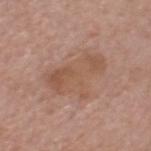{
  "biopsy_status": "not biopsied; imaged during a skin examination",
  "site": "head or neck",
  "patient": {
    "sex": "male",
    "age_approx": 65
  },
  "image": {
    "source": "total-body photography crop",
    "field_of_view_mm": 15
  }
}